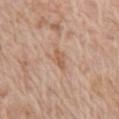| feature | finding |
|---|---|
| notes | total-body-photography surveillance lesion; no biopsy |
| subject | female, approximately 75 years of age |
| image | 15 mm crop, total-body photography |
| location | the arm |